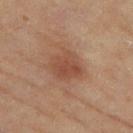Captured under cross-polarized illumination. Longest diameter approximately 4 mm. A female subject approximately 60 years of age. The lesion is located on the left thigh. A 15 mm close-up tile from a total-body photography series done for melanoma screening.